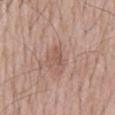Part of a total-body skin-imaging series; this lesion was reviewed on a skin check and was not flagged for biopsy. On the mid back. A close-up tile cropped from a whole-body skin photograph, about 15 mm across. The lesion-visualizer software estimated an area of roughly 3 mm², a shape eccentricity near 0.9, and two-axis asymmetry of about 0.3. The analysis additionally found an average lesion color of about L≈54 a*≈20 b*≈26 (CIELAB), roughly 7 lightness units darker than nearby skin, and a normalized lesion–skin contrast near 5.5. A male subject about 60 years old. This is a white-light tile. The recorded lesion diameter is about 2.5 mm.